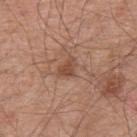The lesion was photographed on a routine skin check and not biopsied; there is no pathology result. The recorded lesion diameter is about 2.5 mm. A male subject about 55 years old. The lesion is on the upper back. A close-up tile cropped from a whole-body skin photograph, about 15 mm across.